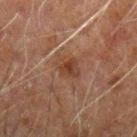Case summary:
• image source — 15 mm crop, total-body photography
• patient — male, aged approximately 60
• site — the arm